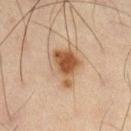{"biopsy_status": "not biopsied; imaged during a skin examination", "image": {"source": "total-body photography crop", "field_of_view_mm": 15}, "lesion_size": {"long_diameter_mm_approx": 4.5}, "patient": {"sex": "male", "age_approx": 45}, "automated_metrics": {"cielab_L": 44, "cielab_a": 16, "cielab_b": 29, "vs_skin_darker_L": 11.0, "vs_skin_contrast_norm": 9.5}, "site": "right thigh", "lighting": "cross-polarized"}